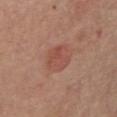follow-up: imaged on a skin check; not biopsied | tile lighting: white-light illumination | image: total-body-photography crop, ~15 mm field of view | automated lesion analysis: an area of roughly 9 mm², a shape eccentricity near 0.65, and two-axis asymmetry of about 0.15; a lesion color around L≈50 a*≈25 b*≈28 in CIELAB, roughly 7 lightness units darker than nearby skin, and a normalized border contrast of about 5.5; an automated nevus-likeness rating near 75 out of 100 | patient: female, approximately 65 years of age | anatomic site: the chest.